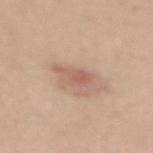Part of a total-body skin-imaging series; this lesion was reviewed on a skin check and was not flagged for biopsy. Located on the back. A female subject, aged approximately 45. A 15 mm close-up tile from a total-body photography series done for melanoma screening.